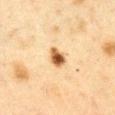Captured during whole-body skin photography for melanoma surveillance; the lesion was not biopsied. The total-body-photography lesion software estimated a mean CIELAB color near L≈50 a*≈19 b*≈36, a lesion–skin lightness drop of about 17, and a normalized lesion–skin contrast near 12. And it measured a border-irregularity rating of about 2/10 and a peripheral color-asymmetry measure near 2. And it measured an automated nevus-likeness rating near 100 out of 100 and a detector confidence of about 100 out of 100 that the crop contains a lesion. A lesion tile, about 15 mm wide, cut from a 3D total-body photograph. The lesion is on the chest. A female patient, in their 40s.